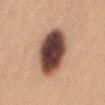Impression: Imaged during a routine full-body skin examination; the lesion was not biopsied and no histopathology is available. Acquisition and patient details: A female subject aged 23 to 27. The lesion is located on the mid back. An algorithmic analysis of the crop reported an automated nevus-likeness rating near 95 out of 100. A lesion tile, about 15 mm wide, cut from a 3D total-body photograph. Imaged with white-light lighting.